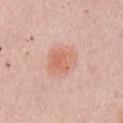biopsy_status: not biopsied; imaged during a skin examination
image:
  source: total-body photography crop
  field_of_view_mm: 15
site: front of the torso
patient:
  sex: female
  age_approx: 65
lesion_size:
  long_diameter_mm_approx: 4.0
automated_metrics:
  nevus_likeness_0_100: 95
lighting: white-light